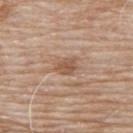workup=catalogued during a skin exam; not biopsied | acquisition=total-body-photography crop, ~15 mm field of view | location=the back | patient=male, roughly 80 years of age.